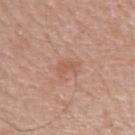biopsy status = catalogued during a skin exam; not biopsied | image = ~15 mm tile from a whole-body skin photo | patient = female, aged 48 to 52 | illumination = white-light | anatomic site = the right forearm | diameter = ≈2.5 mm.